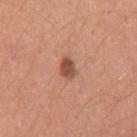– notes — no biopsy performed (imaged during a skin exam)
– automated lesion analysis — a detector confidence of about 100 out of 100 that the crop contains a lesion
– image — total-body-photography crop, ~15 mm field of view
– body site — the arm
– patient — male, in their 30s
– lighting — white-light